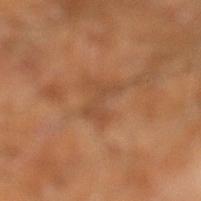{
  "biopsy_status": "not biopsied; imaged during a skin examination",
  "lighting": "cross-polarized",
  "site": "leg",
  "automated_metrics": {
    "shape_asymmetry": 0.3,
    "cielab_L": 45,
    "cielab_a": 21,
    "cielab_b": 33,
    "vs_skin_darker_L": 6.0,
    "border_irregularity_0_10": 6.5,
    "peripheral_color_asymmetry": 0.0
  },
  "image": {
    "source": "total-body photography crop",
    "field_of_view_mm": 15
  },
  "patient": {
    "sex": "male",
    "age_approx": 60
  },
  "lesion_size": {
    "long_diameter_mm_approx": 3.5
  }
}